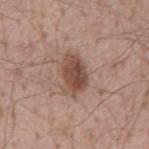The lesion was photographed on a routine skin check and not biopsied; there is no pathology result. A male subject aged 53 to 57. A region of skin cropped from a whole-body photographic capture, roughly 15 mm wide. About 5 mm across. Located on the mid back. The tile uses white-light illumination. Automated image analysis of the tile measured a symmetry-axis asymmetry near 0.2. And it measured a lesion color around L≈48 a*≈20 b*≈25 in CIELAB, about 12 CIELAB-L* units darker than the surrounding skin, and a normalized border contrast of about 9. And it measured a nevus-likeness score of about 95/100 and a lesion-detection confidence of about 100/100.